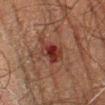<record>
<biopsy_status>not biopsied; imaged during a skin examination</biopsy_status>
<lesion_size>
  <long_diameter_mm_approx>3.5</long_diameter_mm_approx>
</lesion_size>
<lighting>cross-polarized</lighting>
<site>leg</site>
<patient>
  <sex>male</sex>
  <age_approx>80</age_approx>
</patient>
<automated_metrics>
  <cielab_L>26</cielab_L>
  <cielab_a>22</cielab_a>
  <cielab_b>22</cielab_b>
  <vs_skin_darker_L>9.0</vs_skin_darker_L>
  <vs_skin_contrast_norm>9.5</vs_skin_contrast_norm>
  <nevus_likeness_0_100>0</nevus_likeness_0_100>
  <lesion_detection_confidence_0_100>100</lesion_detection_confidence_0_100>
</automated_metrics>
<image>
  <source>total-body photography crop</source>
  <field_of_view_mm>15</field_of_view_mm>
</image>
</record>